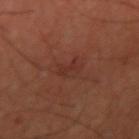Recorded during total-body skin imaging; not selected for excision or biopsy.
An algorithmic analysis of the crop reported a mean CIELAB color near L≈31 a*≈23 b*≈25, roughly 5 lightness units darker than nearby skin, and a normalized border contrast of about 5. It also reported border irregularity of about 3.5 on a 0–10 scale and internal color variation of about 1.5 on a 0–10 scale.
The patient is a male aged 38–42.
On the arm.
This image is a 15 mm lesion crop taken from a total-body photograph.
Approximately 3 mm at its widest.
Imaged with cross-polarized lighting.